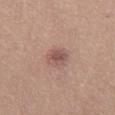Clinical impression: The lesion was photographed on a routine skin check and not biopsied; there is no pathology result. Background: Automated image analysis of the tile measured an area of roughly 5 mm². The software also gave a mean CIELAB color near L≈52 a*≈21 b*≈22, roughly 10 lightness units darker than nearby skin, and a normalized lesion–skin contrast near 7. The software also gave border irregularity of about 2 on a 0–10 scale and internal color variation of about 3 on a 0–10 scale. Captured under white-light illumination. The patient is a male aged 23–27. Cropped from a total-body skin-imaging series; the visible field is about 15 mm. The lesion is on the mid back.